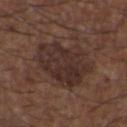Findings:
• workup: total-body-photography surveillance lesion; no biopsy
• image: 15 mm crop, total-body photography
• subject: male, in their 50s
• site: the upper back